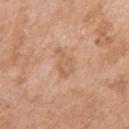Q: Is there a histopathology result?
A: imaged on a skin check; not biopsied
Q: How was the tile lit?
A: white-light illumination
Q: What did automated image analysis measure?
A: a lesion color around L≈61 a*≈21 b*≈34 in CIELAB and a normalized lesion–skin contrast near 5
Q: Who is the patient?
A: female, in their mid-70s
Q: What kind of image is this?
A: 15 mm crop, total-body photography
Q: Lesion location?
A: the right upper arm